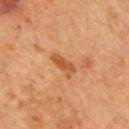Part of a total-body skin-imaging series; this lesion was reviewed on a skin check and was not flagged for biopsy.
The total-body-photography lesion software estimated an area of roughly 4.5 mm², a shape eccentricity near 0.9, and two-axis asymmetry of about 0.25. The analysis additionally found an average lesion color of about L≈49 a*≈25 b*≈39 (CIELAB). It also reported a border-irregularity rating of about 3/10, a color-variation rating of about 1.5/10, and radial color variation of about 0.5. It also reported a classifier nevus-likeness of about 5/100 and a lesion-detection confidence of about 100/100.
The subject is a male aged around 55.
A roughly 15 mm field-of-view crop from a total-body skin photograph.
From the mid back.
Imaged with cross-polarized lighting.